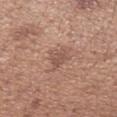A region of skin cropped from a whole-body photographic capture, roughly 15 mm wide. A female subject, aged 28–32. The lesion is located on the left forearm. Imaged with white-light lighting. The recorded lesion diameter is about 3 mm.